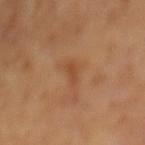Recorded during total-body skin imaging; not selected for excision or biopsy. The lesion-visualizer software estimated a lesion area of about 2.5 mm² and a shape-asymmetry score of about 0.45 (0 = symmetric). The software also gave a mean CIELAB color near L≈45 a*≈23 b*≈34 and a normalized border contrast of about 5.5. And it measured a border-irregularity index near 4.5/10, a within-lesion color-variation index near 0/10, and radial color variation of about 0. This is a cross-polarized tile. The lesion's longest dimension is about 2.5 mm. The patient is a male approximately 65 years of age. A region of skin cropped from a whole-body photographic capture, roughly 15 mm wide.